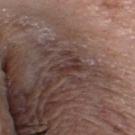workup = total-body-photography surveillance lesion; no biopsy
patient = female, approximately 65 years of age
imaging modality = 15 mm crop, total-body photography
illumination = white-light
site = the head or neck